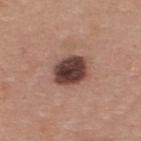| key | value |
|---|---|
| workup | catalogued during a skin exam; not biopsied |
| image | 15 mm crop, total-body photography |
| tile lighting | white-light |
| anatomic site | the upper back |
| automated metrics | an average lesion color of about L≈41 a*≈20 b*≈22 (CIELAB) and roughly 19 lightness units darker than nearby skin; border irregularity of about 1 on a 0–10 scale, a color-variation rating of about 6/10, and radial color variation of about 2 |
| patient | male, aged 33 to 37 |
| lesion size | ~4 mm (longest diameter) |